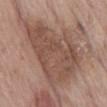Part of a total-body skin-imaging series; this lesion was reviewed on a skin check and was not flagged for biopsy.
A close-up tile cropped from a whole-body skin photograph, about 15 mm across.
About 9 mm across.
Located on the abdomen.
This is a white-light tile.
The subject is a male aged 68–72.